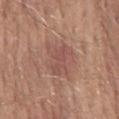Part of a total-body skin-imaging series; this lesion was reviewed on a skin check and was not flagged for biopsy. Automated tile analysis of the lesion measured a border-irregularity rating of about 3.5/10, a within-lesion color-variation index near 3.5/10, and peripheral color asymmetry of about 1. The analysis additionally found a classifier nevus-likeness of about 0/100 and a detector confidence of about 100 out of 100 that the crop contains a lesion. The patient is a male about 55 years old. Captured under white-light illumination. This image is a 15 mm lesion crop taken from a total-body photograph. Approximately 4.5 mm at its widest. The lesion is located on the mid back.